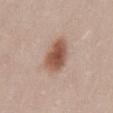The lesion was photographed on a routine skin check and not biopsied; there is no pathology result.
Imaged with white-light lighting.
The lesion is on the abdomen.
Automated image analysis of the tile measured a border-irregularity index near 1.5/10, a within-lesion color-variation index near 4/10, and a peripheral color-asymmetry measure near 1.
A close-up tile cropped from a whole-body skin photograph, about 15 mm across.
A female subject, in their mid- to late 20s.